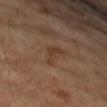Impression: This lesion was catalogued during total-body skin photography and was not selected for biopsy. Acquisition and patient details: An algorithmic analysis of the crop reported a lesion area of about 4 mm² and a symmetry-axis asymmetry near 0.6. The software also gave a mean CIELAB color near L≈38 a*≈18 b*≈29 and about 6 CIELAB-L* units darker than the surrounding skin. The analysis additionally found a border-irregularity index near 6.5/10, internal color variation of about 1.5 on a 0–10 scale, and radial color variation of about 0.5. A region of skin cropped from a whole-body photographic capture, roughly 15 mm wide. On the right forearm. The subject is a female aged around 60.